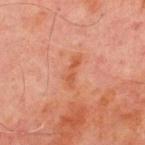  lesion_size:
    long_diameter_mm_approx: 3.5
  patient:
    sex: male
    age_approx: 70
  image:
    source: total-body photography crop
    field_of_view_mm: 15
  site: front of the torso
  lighting: cross-polarized